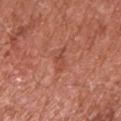| feature | finding |
|---|---|
| workup | no biopsy performed (imaged during a skin exam) |
| patient | male, approximately 65 years of age |
| acquisition | ~15 mm crop, total-body skin-cancer survey |
| location | the left upper arm |
| size | ~3.5 mm (longest diameter) |
| illumination | white-light |
| automated lesion analysis | border irregularity of about 4.5 on a 0–10 scale, internal color variation of about 0 on a 0–10 scale, and peripheral color asymmetry of about 0 |